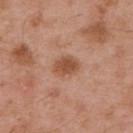Assessment: This lesion was catalogued during total-body skin photography and was not selected for biopsy. Clinical summary: The lesion is on the upper back. This image is a 15 mm lesion crop taken from a total-body photograph. The patient is a male aged around 55.